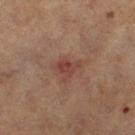| feature | finding |
|---|---|
| follow-up | no biopsy performed (imaged during a skin exam) |
| anatomic site | the left thigh |
| image | 15 mm crop, total-body photography |
| subject | female, aged approximately 60 |
| illumination | cross-polarized |
| TBP lesion metrics | a footprint of about 4 mm² and a shape-asymmetry score of about 0.45 (0 = symmetric) |